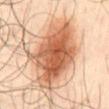This lesion was catalogued during total-body skin photography and was not selected for biopsy.
Captured under cross-polarized illumination.
A male patient, aged 63–67.
Approximately 9 mm at its widest.
This image is a 15 mm lesion crop taken from a total-body photograph.
The lesion is located on the front of the torso.